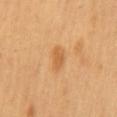The lesion was photographed on a routine skin check and not biopsied; there is no pathology result. A female subject aged 58–62. Approximately 3 mm at its widest. On the mid back. A 15 mm crop from a total-body photograph taken for skin-cancer surveillance. Captured under cross-polarized illumination. Automated tile analysis of the lesion measured an area of roughly 4.5 mm². The analysis additionally found a nevus-likeness score of about 75/100 and a detector confidence of about 100 out of 100 that the crop contains a lesion.